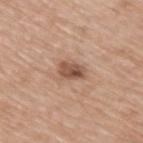The lesion was photographed on a routine skin check and not biopsied; there is no pathology result. The tile uses white-light illumination. A male subject, aged 63–67. The lesion is on the upper back. The lesion's longest dimension is about 3 mm. A close-up tile cropped from a whole-body skin photograph, about 15 mm across.